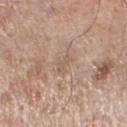workup: catalogued during a skin exam; not biopsied | image: 15 mm crop, total-body photography | site: the left lower leg | tile lighting: white-light illumination | size: ~2.5 mm (longest diameter) | subject: male, in their 80s | automated metrics: a shape eccentricity near 0.8 and a shape-asymmetry score of about 0.45 (0 = symmetric); a border-irregularity rating of about 4.5/10, internal color variation of about 0 on a 0–10 scale, and peripheral color asymmetry of about 0.